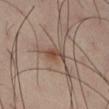Imaged during a routine full-body skin examination; the lesion was not biopsied and no histopathology is available.
The subject is a male aged around 40.
The lesion's longest dimension is about 3 mm.
The lesion is located on the right thigh.
A roughly 15 mm field-of-view crop from a total-body skin photograph.
Imaged with cross-polarized lighting.